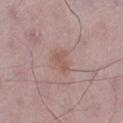Impression: This lesion was catalogued during total-body skin photography and was not selected for biopsy. Context: A male subject aged 48–52. On the left lower leg. The recorded lesion diameter is about 2.5 mm. Automated image analysis of the tile measured border irregularity of about 3.5 on a 0–10 scale, internal color variation of about 1.5 on a 0–10 scale, and radial color variation of about 0.5. A roughly 15 mm field-of-view crop from a total-body skin photograph.